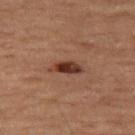The lesion was tiled from a total-body skin photograph and was not biopsied. Captured under cross-polarized illumination. The lesion's longest dimension is about 3.5 mm. Automated tile analysis of the lesion measured a lesion area of about 4.5 mm² and a shape eccentricity near 0.85. It also reported a mean CIELAB color near L≈26 a*≈18 b*≈21, roughly 11 lightness units darker than nearby skin, and a normalized lesion–skin contrast near 11. It also reported an automated nevus-likeness rating near 90 out of 100 and a detector confidence of about 100 out of 100 that the crop contains a lesion. A 15 mm close-up extracted from a 3D total-body photography capture. The patient is a male approximately 70 years of age. From the left thigh.